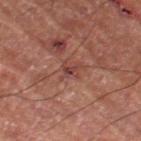This lesion was catalogued during total-body skin photography and was not selected for biopsy. Imaged with cross-polarized lighting. The lesion is on the right thigh. About 2.5 mm across. A lesion tile, about 15 mm wide, cut from a 3D total-body photograph. A male patient, approximately 55 years of age.